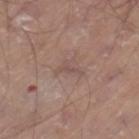The lesion was photographed on a routine skin check and not biopsied; there is no pathology result.
The total-body-photography lesion software estimated a footprint of about 2.5 mm², an eccentricity of roughly 0.9, and two-axis asymmetry of about 0.5. It also reported an average lesion color of about L≈50 a*≈17 b*≈22 (CIELAB) and a normalized border contrast of about 5. It also reported a border-irregularity index near 5.5/10 and internal color variation of about 0 on a 0–10 scale.
This image is a 15 mm lesion crop taken from a total-body photograph.
The recorded lesion diameter is about 3 mm.
The patient is a male aged approximately 80.
Captured under white-light illumination.
From the left thigh.